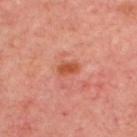Q: Was this lesion biopsied?
A: catalogued during a skin exam; not biopsied
Q: What did automated image analysis measure?
A: a border-irregularity rating of about 1.5/10, internal color variation of about 2 on a 0–10 scale, and radial color variation of about 0.5; a lesion-detection confidence of about 100/100
Q: What is the anatomic site?
A: the upper back
Q: What is the imaging modality?
A: 15 mm crop, total-body photography
Q: What are the patient's age and sex?
A: aged approximately 55
Q: Lesion size?
A: ~2.5 mm (longest diameter)